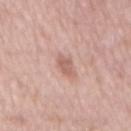biopsy status=total-body-photography surveillance lesion; no biopsy | illumination=white-light illumination | site=the mid back | lesion size=≈3 mm | subject=male, aged around 65 | acquisition=15 mm crop, total-body photography.